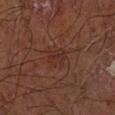This lesion was catalogued during total-body skin photography and was not selected for biopsy.
The lesion is located on the left forearm.
A 15 mm close-up tile from a total-body photography series done for melanoma screening.
The lesion-visualizer software estimated an eccentricity of roughly 0.65. The software also gave border irregularity of about 2.5 on a 0–10 scale and a within-lesion color-variation index near 4.5/10. It also reported a nevus-likeness score of about 0/100 and lesion-presence confidence of about 100/100.
This is a cross-polarized tile.
A male patient, about 70 years old.